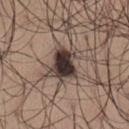notes=total-body-photography surveillance lesion; no biopsy | image source=15 mm crop, total-body photography | patient=male, approximately 65 years of age | anatomic site=the leg | tile lighting=white-light.